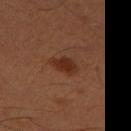notes: total-body-photography surveillance lesion; no biopsy
subject: male, about 50 years old
lighting: cross-polarized
body site: the left thigh
image source: ~15 mm tile from a whole-body skin photo
lesion size: ~3.5 mm (longest diameter)